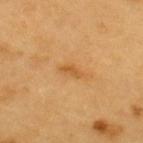{"biopsy_status": "not biopsied; imaged during a skin examination", "patient": {"sex": "male", "age_approx": 60}, "lesion_size": {"long_diameter_mm_approx": 2.5}, "site": "back", "image": {"source": "total-body photography crop", "field_of_view_mm": 15}}